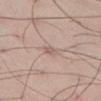No biopsy was performed on this lesion — it was imaged during a full skin examination and was not determined to be concerning.
The lesion is on the abdomen.
A 15 mm crop from a total-body photograph taken for skin-cancer surveillance.
A male patient approximately 45 years of age.